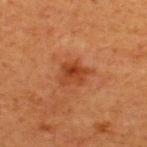Q: Is there a histopathology result?
A: total-body-photography surveillance lesion; no biopsy
Q: How was the tile lit?
A: cross-polarized illumination
Q: What kind of image is this?
A: 15 mm crop, total-body photography
Q: What did automated image analysis measure?
A: a shape eccentricity near 0.6 and a symmetry-axis asymmetry near 0.4; a mean CIELAB color near L≈39 a*≈27 b*≈36, about 9 CIELAB-L* units darker than the surrounding skin, and a normalized lesion–skin contrast near 7.5; a border-irregularity index near 4/10, internal color variation of about 5 on a 0–10 scale, and radial color variation of about 2; an automated nevus-likeness rating near 65 out of 100 and lesion-presence confidence of about 100/100
Q: Lesion size?
A: ~3.5 mm (longest diameter)
Q: What are the patient's age and sex?
A: male, aged around 55
Q: What is the anatomic site?
A: the upper back